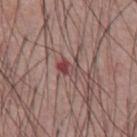  biopsy_status: not biopsied; imaged during a skin examination
  site: abdomen
  lighting: white-light
  image:
    source: total-body photography crop
    field_of_view_mm: 15
  lesion_size:
    long_diameter_mm_approx: 3.0
  patient:
    sex: male
    age_approx: 55